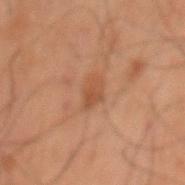biopsy status — catalogued during a skin exam; not biopsied
location — the mid back
automated lesion analysis — a footprint of about 4.5 mm², a shape eccentricity near 0.8, and a symmetry-axis asymmetry near 0.25; a lesion–skin lightness drop of about 6
imaging modality — total-body-photography crop, ~15 mm field of view
tile lighting — cross-polarized
lesion size — ~3 mm (longest diameter)
subject — male, approximately 60 years of age